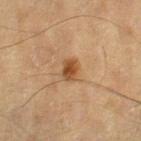notes: catalogued during a skin exam; not biopsied
imaging modality: total-body-photography crop, ~15 mm field of view
subject: male, in their mid-80s or older
body site: the left thigh
TBP lesion metrics: an outline eccentricity of about 0.75 (0 = round, 1 = elongated) and a shape-asymmetry score of about 0.25 (0 = symmetric); a mean CIELAB color near L≈43 a*≈19 b*≈33 and about 10 CIELAB-L* units darker than the surrounding skin; a classifier nevus-likeness of about 95/100 and lesion-presence confidence of about 100/100
illumination: cross-polarized illumination
lesion size: ~2.5 mm (longest diameter)